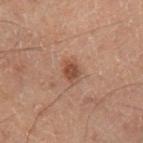Notes:
- biopsy status — catalogued during a skin exam; not biopsied
- subject — male, roughly 70 years of age
- imaging modality — total-body-photography crop, ~15 mm field of view
- body site — the leg